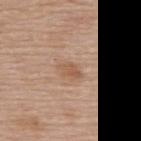No biopsy was performed on this lesion — it was imaged during a full skin examination and was not determined to be concerning.
This image is a 15 mm lesion crop taken from a total-body photograph.
The lesion's longest dimension is about 3 mm.
Located on the back.
Automated tile analysis of the lesion measured an outline eccentricity of about 0.8 (0 = round, 1 = elongated) and a shape-asymmetry score of about 0.3 (0 = symmetric). It also reported a border-irregularity rating of about 3/10 and a color-variation rating of about 1.5/10.
The patient is a female about 60 years old.